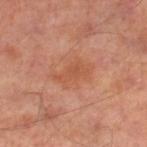Assessment: Recorded during total-body skin imaging; not selected for excision or biopsy. Image and clinical context: Imaged with cross-polarized lighting. This image is a 15 mm lesion crop taken from a total-body photograph. The lesion's longest dimension is about 4 mm. The patient is a male approximately 65 years of age. Located on the right lower leg.